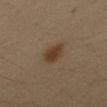Part of a total-body skin-imaging series; this lesion was reviewed on a skin check and was not flagged for biopsy.
About 3 mm across.
The subject is a female about 35 years old.
A region of skin cropped from a whole-body photographic capture, roughly 15 mm wide.
The lesion is on the right forearm.
Imaged with cross-polarized lighting.
Automated image analysis of the tile measured a border-irregularity rating of about 2.5/10, internal color variation of about 2.5 on a 0–10 scale, and radial color variation of about 1.